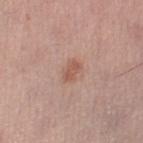Imaged during a routine full-body skin examination; the lesion was not biopsied and no histopathology is available. A 15 mm close-up extracted from a 3D total-body photography capture. A female subject, aged around 50. Located on the right lower leg.